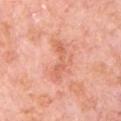Recorded during total-body skin imaging; not selected for excision or biopsy. The total-body-photography lesion software estimated a lesion area of about 5 mm², an outline eccentricity of about 0.95 (0 = round, 1 = elongated), and a symmetry-axis asymmetry near 0.55. The lesion is located on the chest. A 15 mm close-up extracted from a 3D total-body photography capture. Longest diameter approximately 4.5 mm. The subject is a male aged around 80.